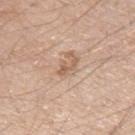notes = imaged on a skin check; not biopsied | TBP lesion metrics = a footprint of about 2.5 mm² and two-axis asymmetry of about 0.5; a border-irregularity index near 6.5/10 and peripheral color asymmetry of about 0 | image source = ~15 mm tile from a whole-body skin photo | subject = male, aged approximately 60 | site = the left upper arm | size = ≈3 mm | tile lighting = white-light illumination.